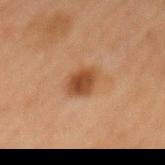- workup: catalogued during a skin exam; not biopsied
- site: the mid back
- acquisition: ~15 mm crop, total-body skin-cancer survey
- subject: male, about 65 years old